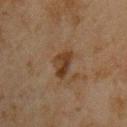<case>
  <biopsy_status>not biopsied; imaged during a skin examination</biopsy_status>
  <image>
    <source>total-body photography crop</source>
    <field_of_view_mm>15</field_of_view_mm>
  </image>
  <patient>
    <sex>male</sex>
    <age_approx>45</age_approx>
  </patient>
  <lighting>cross-polarized</lighting>
  <lesion_size>
    <long_diameter_mm_approx>3.5</long_diameter_mm_approx>
  </lesion_size>
  <site>front of the torso</site>
</case>